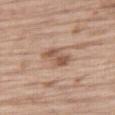workup: total-body-photography surveillance lesion; no biopsy
site: the right thigh
imaging modality: ~15 mm tile from a whole-body skin photo
patient: male, in their 70s